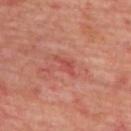{"biopsy_status": "not biopsied; imaged during a skin examination"}